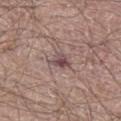| field | value |
|---|---|
| biopsy status | imaged on a skin check; not biopsied |
| image-analysis metrics | a lesion color around L≈48 a*≈18 b*≈17 in CIELAB, about 11 CIELAB-L* units darker than the surrounding skin, and a normalized lesion–skin contrast near 8.5; a border-irregularity index near 3/10, internal color variation of about 5.5 on a 0–10 scale, and peripheral color asymmetry of about 1.5 |
| image | ~15 mm crop, total-body skin-cancer survey |
| size | about 2.5 mm |
| tile lighting | white-light |
| anatomic site | the left thigh |
| subject | male, about 65 years old |